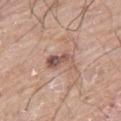Context:
A male patient approximately 60 years of age. Automated tile analysis of the lesion measured a border-irregularity rating of about 4.5/10, internal color variation of about 7 on a 0–10 scale, and radial color variation of about 2. And it measured a classifier nevus-likeness of about 0/100 and lesion-presence confidence of about 100/100. Captured under white-light illumination. Longest diameter approximately 3.5 mm. Located on the right thigh. A close-up tile cropped from a whole-body skin photograph, about 15 mm across.